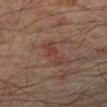Clinical summary:
The recorded lesion diameter is about 4 mm. From the left forearm. The total-body-photography lesion software estimated an area of roughly 5.5 mm², a shape eccentricity near 0.9, and two-axis asymmetry of about 0.35. And it measured a lesion color around L≈33 a*≈17 b*≈21 in CIELAB and about 5 CIELAB-L* units darker than the surrounding skin. A male subject, aged approximately 45. A 15 mm crop from a total-body photograph taken for skin-cancer surveillance. The tile uses cross-polarized illumination.